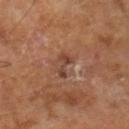Captured during whole-body skin photography for melanoma surveillance; the lesion was not biopsied.
A male subject, aged approximately 65.
A 15 mm crop from a total-body photograph taken for skin-cancer surveillance.
The tile uses cross-polarized illumination.
An algorithmic analysis of the crop reported a lesion area of about 3.5 mm², an eccentricity of roughly 0.9, and two-axis asymmetry of about 0.4. The analysis additionally found an average lesion color of about L≈41 a*≈21 b*≈28 (CIELAB). And it measured internal color variation of about 1.5 on a 0–10 scale and peripheral color asymmetry of about 0.5. The analysis additionally found a classifier nevus-likeness of about 0/100 and lesion-presence confidence of about 100/100.
Longest diameter approximately 3 mm.